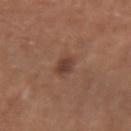This lesion was catalogued during total-body skin photography and was not selected for biopsy. About 2.5 mm across. This image is a 15 mm lesion crop taken from a total-body photograph. A female patient aged 63–67. On the right upper arm. The tile uses white-light illumination.